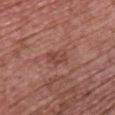Clinical impression: The lesion was photographed on a routine skin check and not biopsied; there is no pathology result. Acquisition and patient details: From the front of the torso. This is a white-light tile. The recorded lesion diameter is about 2.5 mm. A 15 mm close-up extracted from a 3D total-body photography capture. A female subject roughly 65 years of age. Automated image analysis of the tile measured a border-irregularity rating of about 2/10 and peripheral color asymmetry of about 1. The software also gave a nevus-likeness score of about 0/100 and lesion-presence confidence of about 100/100.